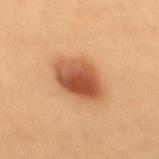Imaged during a routine full-body skin examination; the lesion was not biopsied and no histopathology is available.
On the mid back.
This is a cross-polarized tile.
A 15 mm crop from a total-body photograph taken for skin-cancer surveillance.
The patient is a female aged around 25.
The recorded lesion diameter is about 5 mm.
The lesion-visualizer software estimated a mean CIELAB color near L≈53 a*≈26 b*≈37 and roughly 15 lightness units darker than nearby skin.